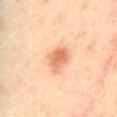biopsy_status: not biopsied; imaged during a skin examination
automated_metrics:
  eccentricity: 0.75
  shape_asymmetry: 0.2
  cielab_L: 66
  cielab_a: 26
  cielab_b: 37
  vs_skin_darker_L: 12.0
  vs_skin_contrast_norm: 7.5
  lesion_detection_confidence_0_100: 100
patient:
  sex: male
  age_approx: 65
image:
  source: total-body photography crop
  field_of_view_mm: 15
lesion_size:
  long_diameter_mm_approx: 3.5
site: lower back
lighting: cross-polarized